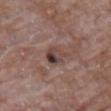Impression: The lesion was tiled from a total-body skin photograph and was not biopsied. Context: The lesion is on the chest. Longest diameter approximately 3.5 mm. Cropped from a whole-body photographic skin survey; the tile spans about 15 mm. A male subject, aged approximately 65. The lesion-visualizer software estimated a border-irregularity index near 3/10, internal color variation of about 10 on a 0–10 scale, and a peripheral color-asymmetry measure near 4.5. The tile uses white-light illumination.